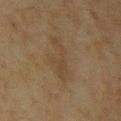biopsy status: total-body-photography surveillance lesion; no biopsy
imaging modality: 15 mm crop, total-body photography
patient: female, aged approximately 55
body site: the arm